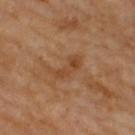workup: no biopsy performed (imaged during a skin exam)
illumination: cross-polarized
automated metrics: a lesion color around L≈46 a*≈22 b*≈36 in CIELAB, about 7 CIELAB-L* units darker than the surrounding skin, and a normalized border contrast of about 6; a nevus-likeness score of about 0/100 and a lesion-detection confidence of about 100/100
acquisition: total-body-photography crop, ~15 mm field of view
diameter: ~4 mm (longest diameter)
site: the upper back